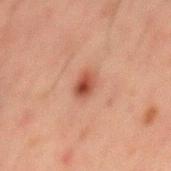Assessment: This lesion was catalogued during total-body skin photography and was not selected for biopsy. Clinical summary: Measured at roughly 2.5 mm in maximum diameter. Automated image analysis of the tile measured an eccentricity of roughly 0.7 and a shape-asymmetry score of about 0.15 (0 = symmetric). The analysis additionally found a lesion color around L≈39 a*≈22 b*≈26 in CIELAB. It also reported a border-irregularity index near 1.5/10, a within-lesion color-variation index near 6/10, and peripheral color asymmetry of about 2.5. On the back. This is a cross-polarized tile. A roughly 15 mm field-of-view crop from a total-body skin photograph. A male subject, aged 48–52.